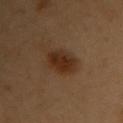Assessment:
The lesion was photographed on a routine skin check and not biopsied; there is no pathology result.
Background:
The lesion-visualizer software estimated a lesion color around L≈25 a*≈16 b*≈26 in CIELAB and a lesion–skin lightness drop of about 8. It also reported a border-irregularity rating of about 2/10, a within-lesion color-variation index near 3.5/10, and radial color variation of about 1. The software also gave a detector confidence of about 100 out of 100 that the crop contains a lesion. From the front of the torso. This is a cross-polarized tile. The lesion's longest dimension is about 4.5 mm. A close-up tile cropped from a whole-body skin photograph, about 15 mm across. The subject is a female aged approximately 60.